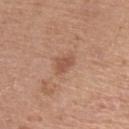The lesion was photographed on a routine skin check and not biopsied; there is no pathology result.
The recorded lesion diameter is about 2.5 mm.
A male subject approximately 55 years of age.
A lesion tile, about 15 mm wide, cut from a 3D total-body photograph.
The lesion is located on the arm.
Imaged with white-light lighting.
An algorithmic analysis of the crop reported a lesion–skin lightness drop of about 9 and a normalized border contrast of about 6.5.